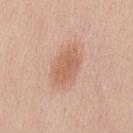Clinical impression:
Recorded during total-body skin imaging; not selected for excision or biopsy.
Context:
A male subject, aged around 40. From the mid back. The recorded lesion diameter is about 5 mm. Imaged with white-light lighting. A 15 mm close-up extracted from a 3D total-body photography capture.